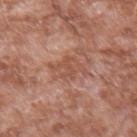No biopsy was performed on this lesion — it was imaged during a full skin examination and was not determined to be concerning.